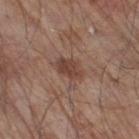{"lesion_size": {"long_diameter_mm_approx": 3.5}, "site": "right thigh", "image": {"source": "total-body photography crop", "field_of_view_mm": 15}, "patient": {"sex": "male", "age_approx": 80}, "lighting": "white-light", "automated_metrics": {"nevus_likeness_0_100": 0, "lesion_detection_confidence_0_100": 100}}